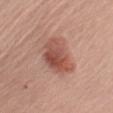workup: catalogued during a skin exam; not biopsied | lighting: white-light | subject: female, roughly 65 years of age | imaging modality: ~15 mm tile from a whole-body skin photo | automated lesion analysis: two-axis asymmetry of about 0.25; peripheral color asymmetry of about 2 | lesion size: about 5 mm | location: the left upper arm.